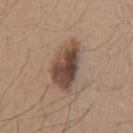workup = catalogued during a skin exam; not biopsied
subject = male, in their mid- to late 20s
image = total-body-photography crop, ~15 mm field of view
size = ≈6 mm
lighting = white-light
TBP lesion metrics = an area of roughly 15 mm², an eccentricity of roughly 0.85, and two-axis asymmetry of about 0.25; a mean CIELAB color near L≈46 a*≈16 b*≈25, about 15 CIELAB-L* units darker than the surrounding skin, and a normalized lesion–skin contrast near 11; a border-irregularity rating of about 3/10, internal color variation of about 7.5 on a 0–10 scale, and peripheral color asymmetry of about 2.5
site = the chest